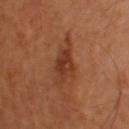imaging modality=~15 mm tile from a whole-body skin photo; lesion size=about 5.5 mm; subject=male, approximately 40 years of age; site=the upper back; lighting=cross-polarized illumination.